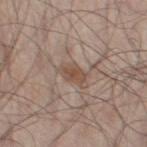Q: Who is the patient?
A: male, approximately 55 years of age
Q: How large is the lesion?
A: ~3 mm (longest diameter)
Q: How was the tile lit?
A: white-light illumination
Q: What did automated image analysis measure?
A: a lesion color around L≈50 a*≈17 b*≈26 in CIELAB and about 9 CIELAB-L* units darker than the surrounding skin; a border-irregularity index near 2/10, internal color variation of about 2 on a 0–10 scale, and peripheral color asymmetry of about 0.5; a classifier nevus-likeness of about 30/100
Q: What is the imaging modality?
A: total-body-photography crop, ~15 mm field of view
Q: Lesion location?
A: the left thigh